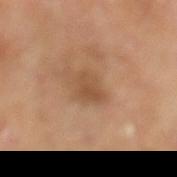body site — the leg
diameter — ~2.5 mm (longest diameter)
lighting — cross-polarized
image — ~15 mm crop, total-body skin-cancer survey
subject — male, aged approximately 65